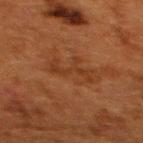Imaged during a routine full-body skin examination; the lesion was not biopsied and no histopathology is available.
The subject is a female in their 50s.
The lesion's longest dimension is about 4.5 mm.
On the upper back.
A 15 mm crop from a total-body photograph taken for skin-cancer surveillance.